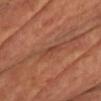Q: Was a biopsy performed?
A: imaged on a skin check; not biopsied
Q: What is the anatomic site?
A: the chest
Q: Patient demographics?
A: roughly 55 years of age
Q: What is the imaging modality?
A: ~15 mm crop, total-body skin-cancer survey